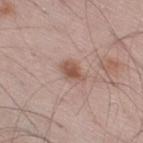follow-up — catalogued during a skin exam; not biopsied
lighting — white-light illumination
image — total-body-photography crop, ~15 mm field of view
size — ~3 mm (longest diameter)
site — the right thigh
subject — male, aged 53 to 57